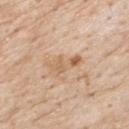Clinical impression:
Captured during whole-body skin photography for melanoma surveillance; the lesion was not biopsied.
Clinical summary:
A male subject aged around 85 or older. A lesion tile, about 15 mm wide, cut from a 3D total-body photograph. The lesion is located on the upper back. About 4 mm across. This is a white-light tile.